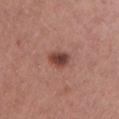Clinical impression: This lesion was catalogued during total-body skin photography and was not selected for biopsy. Clinical summary: From the chest. About 3 mm across. A female subject, aged around 25. A region of skin cropped from a whole-body photographic capture, roughly 15 mm wide. This is a white-light tile.